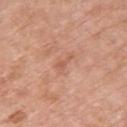Part of a total-body skin-imaging series; this lesion was reviewed on a skin check and was not flagged for biopsy. The recorded lesion diameter is about 3 mm. A 15 mm crop from a total-body photograph taken for skin-cancer surveillance. A female patient, in their mid-60s. Captured under white-light illumination. The lesion-visualizer software estimated an area of roughly 2.5 mm², an outline eccentricity of about 0.9 (0 = round, 1 = elongated), and a symmetry-axis asymmetry near 0.6. It also reported a classifier nevus-likeness of about 0/100 and a detector confidence of about 100 out of 100 that the crop contains a lesion. On the upper back.